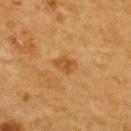follow-up = catalogued during a skin exam; not biopsied | body site = the back | subject = female, aged around 55 | automated metrics = a footprint of about 4.5 mm², a shape eccentricity near 0.75, and two-axis asymmetry of about 0.25; an average lesion color of about L≈54 a*≈23 b*≈46 (CIELAB) and a normalized lesion–skin contrast near 6.5; a border-irregularity rating of about 2.5/10, a color-variation rating of about 2.5/10, and radial color variation of about 1; an automated nevus-likeness rating near 30 out of 100 and lesion-presence confidence of about 100/100 | lesion diameter = ≈3 mm | imaging modality = total-body-photography crop, ~15 mm field of view | tile lighting = cross-polarized.